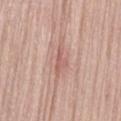biopsy status: catalogued during a skin exam; not biopsied
automated metrics: a mean CIELAB color near L≈60 a*≈22 b*≈25 and a lesion–skin lightness drop of about 9; a color-variation rating of about 1.5/10 and peripheral color asymmetry of about 0.5
subject: female, in their mid- to late 60s
lesion size: ~5 mm (longest diameter)
image: ~15 mm crop, total-body skin-cancer survey
body site: the lower back